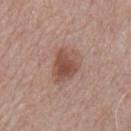Assessment:
The lesion was photographed on a routine skin check and not biopsied; there is no pathology result.
Image and clinical context:
The patient is a male aged 73–77. A lesion tile, about 15 mm wide, cut from a 3D total-body photograph. The tile uses white-light illumination. From the back. Approximately 4.5 mm at its widest. The total-body-photography lesion software estimated an average lesion color of about L≈50 a*≈21 b*≈26 (CIELAB), a lesion–skin lightness drop of about 11, and a normalized border contrast of about 8. The analysis additionally found a border-irregularity index near 2.5/10 and a within-lesion color-variation index near 4.5/10. The software also gave a classifier nevus-likeness of about 90/100 and a lesion-detection confidence of about 100/100.